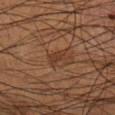The lesion was photographed on a routine skin check and not biopsied; there is no pathology result.
Cropped from a total-body skin-imaging series; the visible field is about 15 mm.
The subject is a male roughly 55 years of age.
On the left lower leg.